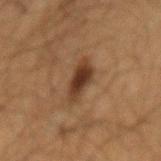Case summary:
- follow-up: total-body-photography surveillance lesion; no biopsy